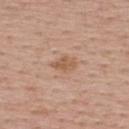Notes:
– subject: female, aged approximately 55
– body site: the back
– size: ~3 mm (longest diameter)
– imaging modality: ~15 mm crop, total-body skin-cancer survey
– illumination: white-light illumination
– automated metrics: a border-irregularity index near 2.5/10 and a color-variation rating of about 2/10; a classifier nevus-likeness of about 0/100 and a detector confidence of about 100 out of 100 that the crop contains a lesion